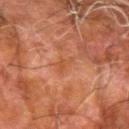Impression: This lesion was catalogued during total-body skin photography and was not selected for biopsy. Context: The lesion-visualizer software estimated an area of roughly 2.5 mm², an eccentricity of roughly 0.9, and a symmetry-axis asymmetry near 0.5. This is a cross-polarized tile. From the left forearm. Cropped from a whole-body photographic skin survey; the tile spans about 15 mm. A male subject about 80 years old.